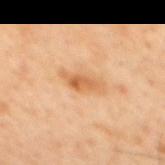Part of a total-body skin-imaging series; this lesion was reviewed on a skin check and was not flagged for biopsy. Automated image analysis of the tile measured a border-irregularity index near 4/10 and peripheral color asymmetry of about 1.5. This is a cross-polarized tile. The recorded lesion diameter is about 4 mm. From the mid back. A 15 mm crop from a total-body photograph taken for skin-cancer surveillance. A male patient, aged 43–47.